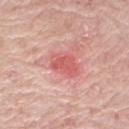Findings:
• workup: no biopsy performed (imaged during a skin exam)
• patient: female, aged approximately 70
• image: total-body-photography crop, ~15 mm field of view
• automated metrics: a footprint of about 7.5 mm², an eccentricity of roughly 0.65, and a shape-asymmetry score of about 0.3 (0 = symmetric); an average lesion color of about L≈60 a*≈34 b*≈26 (CIELAB); border irregularity of about 2.5 on a 0–10 scale, internal color variation of about 2 on a 0–10 scale, and peripheral color asymmetry of about 0.5
• lighting: white-light illumination
• diameter: ~3.5 mm (longest diameter)
• site: the left upper arm